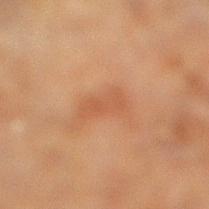workup: total-body-photography surveillance lesion; no biopsy
image-analysis metrics: a mean CIELAB color near L≈45 a*≈21 b*≈31, a lesion–skin lightness drop of about 6, and a normalized border contrast of about 5; a within-lesion color-variation index near 1.5/10; a classifier nevus-likeness of about 0/100
patient: male, about 70 years old
lighting: cross-polarized illumination
lesion diameter: about 3.5 mm
anatomic site: the right lower leg
imaging modality: ~15 mm crop, total-body skin-cancer survey